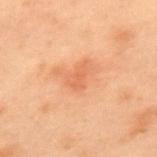Assessment:
The lesion was photographed on a routine skin check and not biopsied; there is no pathology result.
Acquisition and patient details:
The recorded lesion diameter is about 2.5 mm. Cropped from a total-body skin-imaging series; the visible field is about 15 mm. On the upper back. Captured under cross-polarized illumination. Automated tile analysis of the lesion measured a mean CIELAB color near L≈52 a*≈24 b*≈34, roughly 6 lightness units darker than nearby skin, and a normalized border contrast of about 4.5. The analysis additionally found border irregularity of about 3.5 on a 0–10 scale. A male subject in their mid- to late 50s.